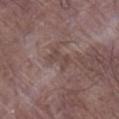Impression: No biopsy was performed on this lesion — it was imaged during a full skin examination and was not determined to be concerning. Clinical summary: A male patient aged 78 to 82. Measured at roughly 3 mm in maximum diameter. An algorithmic analysis of the crop reported a footprint of about 4.5 mm², an outline eccentricity of about 0.85 (0 = round, 1 = elongated), and two-axis asymmetry of about 0.3. It also reported a detector confidence of about 60 out of 100 that the crop contains a lesion. The tile uses white-light illumination. A 15 mm close-up tile from a total-body photography series done for melanoma screening. The lesion is located on the right lower leg.